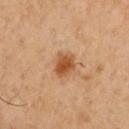Case summary:
- biopsy status: imaged on a skin check; not biopsied
- lighting: cross-polarized
- lesion diameter: about 3 mm
- subject: male, approximately 50 years of age
- image: ~15 mm tile from a whole-body skin photo
- location: the chest
- automated lesion analysis: a footprint of about 6 mm², a shape eccentricity near 0.6, and a shape-asymmetry score of about 0.2 (0 = symmetric); border irregularity of about 2 on a 0–10 scale, a color-variation rating of about 3/10, and a peripheral color-asymmetry measure near 1